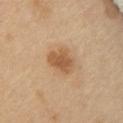Q: Was this lesion biopsied?
A: catalogued during a skin exam; not biopsied
Q: How was this image acquired?
A: ~15 mm tile from a whole-body skin photo
Q: Where on the body is the lesion?
A: the chest
Q: What are the patient's age and sex?
A: female, aged around 55
Q: Automated lesion metrics?
A: a footprint of about 8 mm² and two-axis asymmetry of about 0.25; a border-irregularity index near 2.5/10 and radial color variation of about 1; a classifier nevus-likeness of about 90/100 and a detector confidence of about 100 out of 100 that the crop contains a lesion
Q: Lesion size?
A: ~3.5 mm (longest diameter)